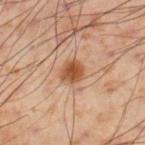notes: imaged on a skin check; not biopsied | illumination: cross-polarized illumination | diameter: ≈3 mm | image-analysis metrics: a footprint of about 6 mm², an outline eccentricity of about 0.25 (0 = round, 1 = elongated), and a symmetry-axis asymmetry near 0.2 | patient: male, aged 53 to 57 | acquisition: 15 mm crop, total-body photography | body site: the right lower leg.